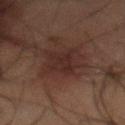| field | value |
|---|---|
| notes | total-body-photography surveillance lesion; no biopsy |
| lighting | cross-polarized illumination |
| anatomic site | the mid back |
| acquisition | total-body-photography crop, ~15 mm field of view |
| subject | male, aged around 50 |
| diameter | ~5 mm (longest diameter) |